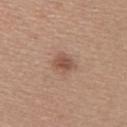Background:
The lesion is located on the upper back. A 15 mm close-up extracted from a 3D total-body photography capture. A female patient aged approximately 30.